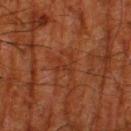site: the left thigh; image: ~15 mm crop, total-body skin-cancer survey; patient: male, in their 80s.